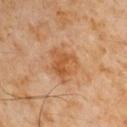{
  "image": {
    "source": "total-body photography crop",
    "field_of_view_mm": 15
  },
  "lesion_size": {
    "long_diameter_mm_approx": 4.5
  },
  "lighting": "cross-polarized",
  "patient": {
    "sex": "male",
    "age_approx": 60
  },
  "site": "arm"
}